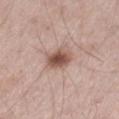Captured during whole-body skin photography for melanoma surveillance; the lesion was not biopsied. Located on the left thigh. A close-up tile cropped from a whole-body skin photograph, about 15 mm across. A male subject, about 55 years old. This is a white-light tile.